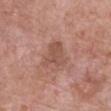Captured during whole-body skin photography for melanoma surveillance; the lesion was not biopsied.
The lesion-visualizer software estimated an area of roughly 7.5 mm², an outline eccentricity of about 0.6 (0 = round, 1 = elongated), and a symmetry-axis asymmetry near 0.5. The analysis additionally found a border-irregularity rating of about 5/10 and a peripheral color-asymmetry measure near 1. The analysis additionally found an automated nevus-likeness rating near 0 out of 100 and lesion-presence confidence of about 100/100.
A 15 mm crop from a total-body photograph taken for skin-cancer surveillance.
The lesion is located on the chest.
The tile uses white-light illumination.
The patient is a female aged 68 to 72.
The recorded lesion diameter is about 4 mm.